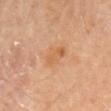Imaged during a routine full-body skin examination; the lesion was not biopsied and no histopathology is available. A region of skin cropped from a whole-body photographic capture, roughly 15 mm wide. From the abdomen. The subject is a male aged around 60.